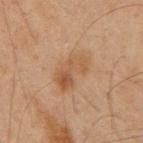Notes:
– biopsy status · no biopsy performed (imaged during a skin exam)
– diameter · ~4.5 mm (longest diameter)
– patient · male, aged 63 to 67
– tile lighting · cross-polarized
– image source · ~15 mm tile from a whole-body skin photo
– location · the mid back
– TBP lesion metrics · a footprint of about 9.5 mm², an outline eccentricity of about 0.85 (0 = round, 1 = elongated), and two-axis asymmetry of about 0.35